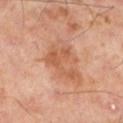Q: What did automated image analysis measure?
A: an area of roughly 9 mm² and an eccentricity of roughly 0.7; an average lesion color of about L≈56 a*≈25 b*≈35 (CIELAB) and a lesion–skin lightness drop of about 8; radial color variation of about 0.5
Q: Who is the patient?
A: male, aged around 50
Q: How large is the lesion?
A: ≈4.5 mm
Q: Illumination type?
A: cross-polarized
Q: What is the imaging modality?
A: 15 mm crop, total-body photography
Q: Where on the body is the lesion?
A: the left lower leg